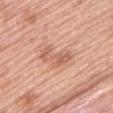| key | value |
|---|---|
| biopsy status | catalogued during a skin exam; not biopsied |
| image | 15 mm crop, total-body photography |
| subject | female, aged 58 to 62 |
| lesion size | ~4.5 mm (longest diameter) |
| tile lighting | white-light |
| site | the upper back |